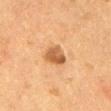From the abdomen. About 3 mm across. Automated tile analysis of the lesion measured a lesion area of about 5.5 mm², an outline eccentricity of about 0.55 (0 = round, 1 = elongated), and a symmetry-axis asymmetry near 0.3. The software also gave a lesion color around L≈47 a*≈21 b*≈34 in CIELAB, about 12 CIELAB-L* units darker than the surrounding skin, and a lesion-to-skin contrast of about 8.5 (normalized; higher = more distinct). It also reported a border-irregularity index near 2.5/10 and a peripheral color-asymmetry measure near 1.5. And it measured an automated nevus-likeness rating near 85 out of 100 and a detector confidence of about 100 out of 100 that the crop contains a lesion. A 15 mm crop from a total-body photograph taken for skin-cancer surveillance. This is a cross-polarized tile. A female patient roughly 50 years of age.